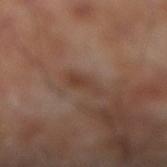Q: Is there a histopathology result?
A: catalogued during a skin exam; not biopsied
Q: Lesion location?
A: the right lower leg
Q: Illumination type?
A: cross-polarized illumination
Q: What is the imaging modality?
A: total-body-photography crop, ~15 mm field of view